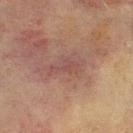Clinical summary:
A close-up tile cropped from a whole-body skin photograph, about 15 mm across. The lesion-visualizer software estimated an area of roughly 4 mm², an outline eccentricity of about 0.9 (0 = round, 1 = elongated), and a shape-asymmetry score of about 0.5 (0 = symmetric). And it measured a normalized border contrast of about 4.5. A male patient, about 70 years old. The lesion is on the front of the torso. Imaged with cross-polarized lighting.